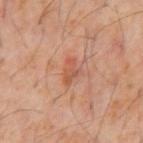The lesion was tiled from a total-body skin photograph and was not biopsied. The tile uses cross-polarized illumination. Longest diameter approximately 3 mm. Cropped from a total-body skin-imaging series; the visible field is about 15 mm. The lesion is on the abdomen. The subject is a male approximately 60 years of age.